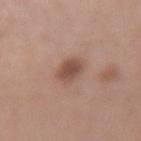This lesion was catalogued during total-body skin photography and was not selected for biopsy. Captured under white-light illumination. The subject is a female aged around 35. On the right forearm. The recorded lesion diameter is about 3 mm. A region of skin cropped from a whole-body photographic capture, roughly 15 mm wide.